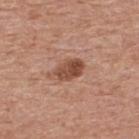Case summary:
– notes: catalogued during a skin exam; not biopsied
– size: about 3.5 mm
– lighting: white-light
– subject: male, aged 78–82
– image: ~15 mm tile from a whole-body skin photo
– location: the back
– image-analysis metrics: a lesion color around L≈48 a*≈23 b*≈29 in CIELAB, roughly 13 lightness units darker than nearby skin, and a normalized border contrast of about 9